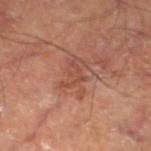<tbp_lesion>
  <biopsy_status>not biopsied; imaged during a skin examination</biopsy_status>
  <site>left thigh</site>
  <lighting>cross-polarized</lighting>
  <image>
    <source>total-body photography crop</source>
    <field_of_view_mm>15</field_of_view_mm>
  </image>
  <lesion_size>
    <long_diameter_mm_approx>4.0</long_diameter_mm_approx>
  </lesion_size>
</tbp_lesion>